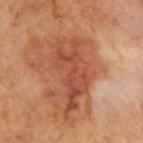<lesion>
  <biopsy_status>not biopsied; imaged during a skin examination</biopsy_status>
  <lighting>cross-polarized</lighting>
  <image>
    <source>total-body photography crop</source>
    <field_of_view_mm>15</field_of_view_mm>
  </image>
  <site>chest</site>
  <patient>
    <sex>male</sex>
    <age_approx>65</age_approx>
  </patient>
  <lesion_size>
    <long_diameter_mm_approx>8.5</long_diameter_mm_approx>
  </lesion_size>
</lesion>